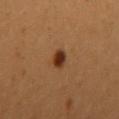Recorded during total-body skin imaging; not selected for excision or biopsy.
The lesion is on the right upper arm.
A 15 mm crop from a total-body photograph taken for skin-cancer surveillance.
Captured under cross-polarized illumination.
Longest diameter approximately 2 mm.
A female patient, in their mid-20s.